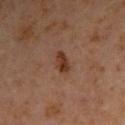– follow-up — imaged on a skin check; not biopsied
– lesion diameter — ≈3 mm
– body site — the right upper arm
– image — 15 mm crop, total-body photography
– subject — male, aged 58 to 62